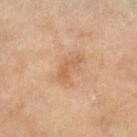<tbp_lesion>
<biopsy_status>not biopsied; imaged during a skin examination</biopsy_status>
<patient>
  <sex>female</sex>
  <age_approx>60</age_approx>
</patient>
<site>right lower leg</site>
<image>
  <source>total-body photography crop</source>
  <field_of_view_mm>15</field_of_view_mm>
</image>
<lesion_size>
  <long_diameter_mm_approx>3.5</long_diameter_mm_approx>
</lesion_size>
<automated_metrics>
  <eccentricity>0.95</eccentricity>
  <shape_asymmetry>0.5</shape_asymmetry>
  <cielab_L>54</cielab_L>
  <cielab_a>19</cielab_a>
  <cielab_b>33</cielab_b>
  <vs_skin_darker_L>7.0</vs_skin_darker_L>
  <vs_skin_contrast_norm>5.5</vs_skin_contrast_norm>
  <color_variation_0_10>0.5</color_variation_0_10>
  <peripheral_color_asymmetry>0.0</peripheral_color_asymmetry>
</automated_metrics>
<lighting>cross-polarized</lighting>
</tbp_lesion>